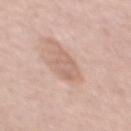Part of a total-body skin-imaging series; this lesion was reviewed on a skin check and was not flagged for biopsy. From the front of the torso. Captured under white-light illumination. A close-up tile cropped from a whole-body skin photograph, about 15 mm across. A male patient, approximately 55 years of age. Automated tile analysis of the lesion measured an area of roughly 8 mm² and a symmetry-axis asymmetry near 0.25. The analysis additionally found a mean CIELAB color near L≈64 a*≈19 b*≈27, a lesion–skin lightness drop of about 8, and a normalized border contrast of about 5.5. The software also gave radial color variation of about 1. Approximately 4.5 mm at its widest.